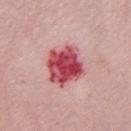The lesion was tiled from a total-body skin photograph and was not biopsied.
On the chest.
A lesion tile, about 15 mm wide, cut from a 3D total-body photograph.
A female patient, about 65 years old.